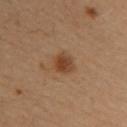Q: Was this lesion biopsied?
A: no biopsy performed (imaged during a skin exam)
Q: Lesion location?
A: the upper back
Q: How was the tile lit?
A: cross-polarized
Q: Patient demographics?
A: female, in their mid- to late 30s
Q: How was this image acquired?
A: total-body-photography crop, ~15 mm field of view
Q: What is the lesion's diameter?
A: ≈2.5 mm
Q: What did automated image analysis measure?
A: a footprint of about 5.5 mm² and a shape-asymmetry score of about 0.2 (0 = symmetric); internal color variation of about 4.5 on a 0–10 scale and a peripheral color-asymmetry measure near 1.5; an automated nevus-likeness rating near 95 out of 100